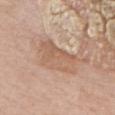The lesion was tiled from a total-body skin photograph and was not biopsied. The tile uses white-light illumination. A 15 mm crop from a total-body photograph taken for skin-cancer surveillance. The total-body-photography lesion software estimated a footprint of about 13 mm², an outline eccentricity of about 0.9 (0 = round, 1 = elongated), and a symmetry-axis asymmetry near 0.25. From the upper back. The subject is a male aged approximately 80. Measured at roughly 6 mm in maximum diameter.